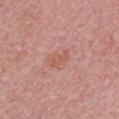Findings:
– notes: imaged on a skin check; not biopsied
– location: the chest
– automated metrics: about 6 CIELAB-L* units darker than the surrounding skin and a normalized border contrast of about 5.5; border irregularity of about 4 on a 0–10 scale, a color-variation rating of about 1/10, and a peripheral color-asymmetry measure near 0; a classifier nevus-likeness of about 20/100
– tile lighting: white-light
– lesion size: ≈3 mm
– imaging modality: 15 mm crop, total-body photography
– patient: female, aged 28 to 32